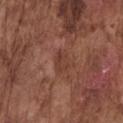Imaged during a routine full-body skin examination; the lesion was not biopsied and no histopathology is available. Automated tile analysis of the lesion measured border irregularity of about 2 on a 0–10 scale and peripheral color asymmetry of about 1. The analysis additionally found a classifier nevus-likeness of about 0/100. A male patient aged around 75. The tile uses white-light illumination. About 2.5 mm across. Cropped from a total-body skin-imaging series; the visible field is about 15 mm. Located on the chest.